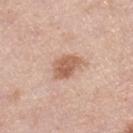Assessment: Captured during whole-body skin photography for melanoma surveillance; the lesion was not biopsied. Clinical summary: A female patient roughly 65 years of age. The lesion is located on the right lower leg. Imaged with white-light lighting. Measured at roughly 4 mm in maximum diameter. This image is a 15 mm lesion crop taken from a total-body photograph.